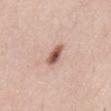Case summary:
- notes: imaged on a skin check; not biopsied
- subject: male, aged 48–52
- image: ~15 mm crop, total-body skin-cancer survey
- location: the chest